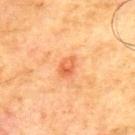Impression:
Captured during whole-body skin photography for melanoma surveillance; the lesion was not biopsied.
Context:
The lesion-visualizer software estimated an area of roughly 4 mm², an eccentricity of roughly 0.7, and a symmetry-axis asymmetry near 0.2. The analysis additionally found a lesion color around L≈53 a*≈28 b*≈38 in CIELAB, a lesion–skin lightness drop of about 9, and a normalized lesion–skin contrast near 6.5. The analysis additionally found a border-irregularity index near 1.5/10, internal color variation of about 4.5 on a 0–10 scale, and a peripheral color-asymmetry measure near 2. The subject is a male in their 70s. Located on the upper back. A 15 mm close-up extracted from a 3D total-body photography capture.